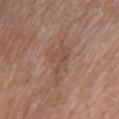Captured during whole-body skin photography for melanoma surveillance; the lesion was not biopsied. A close-up tile cropped from a whole-body skin photograph, about 15 mm across. The lesion is located on the upper back. A female patient, in their 60s.